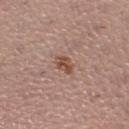image = total-body-photography crop, ~15 mm field of view
patient = female, in their mid-50s
anatomic site = the left lower leg